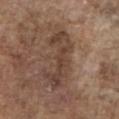Approximately 7.5 mm at its widest.
Captured under white-light illumination.
The subject is a male about 75 years old.
The lesion is located on the chest.
A lesion tile, about 15 mm wide, cut from a 3D total-body photograph.